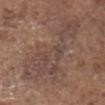The tile uses white-light illumination.
About 11.5 mm across.
A male patient aged 73 to 77.
This image is a 15 mm lesion crop taken from a total-body photograph.
The lesion is on the head or neck.